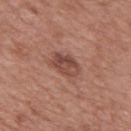No biopsy was performed on this lesion — it was imaged during a full skin examination and was not determined to be concerning. The recorded lesion diameter is about 4 mm. A male patient, approximately 50 years of age. A close-up tile cropped from a whole-body skin photograph, about 15 mm across. The lesion is on the back.